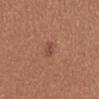The lesion was photographed on a routine skin check and not biopsied; there is no pathology result.
A region of skin cropped from a whole-body photographic capture, roughly 15 mm wide.
This is a white-light tile.
Located on the mid back.
Approximately 2.5 mm at its widest.
The subject is a female aged 23–27.